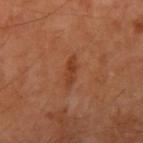Clinical summary:
An algorithmic analysis of the crop reported a border-irregularity index near 2.5/10, a within-lesion color-variation index near 1.5/10, and peripheral color asymmetry of about 0.5. And it measured a classifier nevus-likeness of about 20/100. A lesion tile, about 15 mm wide, cut from a 3D total-body photograph. From the right upper arm. Measured at roughly 3.5 mm in maximum diameter. Captured under cross-polarized illumination. The patient is a male in their mid-60s.